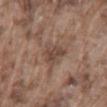A male subject aged around 75. This is a white-light tile. Cropped from a total-body skin-imaging series; the visible field is about 15 mm. Longest diameter approximately 3.5 mm. On the lower back.